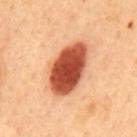Impression: The lesion was tiled from a total-body skin photograph and was not biopsied. Acquisition and patient details: The subject is a male aged approximately 50. On the mid back. A lesion tile, about 15 mm wide, cut from a 3D total-body photograph. The recorded lesion diameter is about 7 mm.